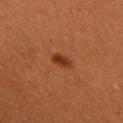The lesion was tiled from a total-body skin photograph and was not biopsied.